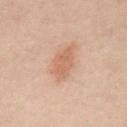  biopsy_status: not biopsied; imaged during a skin examination
  lighting: cross-polarized
  image:
    source: total-body photography crop
    field_of_view_mm: 15
  patient:
    sex: male
    age_approx: 50
  automated_metrics:
    area_mm2_approx: 7.5
    eccentricity: 0.8
    shape_asymmetry: 0.2
    vs_skin_contrast_norm: 6.0
    nevus_likeness_0_100: 90
  site: abdomen